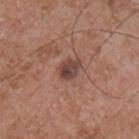Captured during whole-body skin photography for melanoma surveillance; the lesion was not biopsied. About 3 mm across. The lesion is on the chest. The total-body-photography lesion software estimated a lesion area of about 5 mm² and a shape eccentricity near 0.7. It also reported a lesion–skin lightness drop of about 11 and a normalized lesion–skin contrast near 9. It also reported a border-irregularity index near 2/10, a within-lesion color-variation index near 5/10, and peripheral color asymmetry of about 2. The tile uses white-light illumination. A male subject, approximately 55 years of age. A 15 mm close-up extracted from a 3D total-body photography capture.